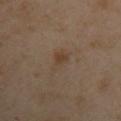Q: Was this lesion biopsied?
A: no biopsy performed (imaged during a skin exam)
Q: What lighting was used for the tile?
A: cross-polarized
Q: What is the anatomic site?
A: the left upper arm
Q: How was this image acquired?
A: ~15 mm crop, total-body skin-cancer survey
Q: What are the patient's age and sex?
A: female, aged 38 to 42
Q: Lesion size?
A: ≈3.5 mm